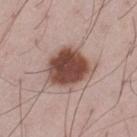The lesion was photographed on a routine skin check and not biopsied; there is no pathology result.
A male patient approximately 50 years of age.
On the leg.
A roughly 15 mm field-of-view crop from a total-body skin photograph.
An algorithmic analysis of the crop reported an eccentricity of roughly 0.6 and a symmetry-axis asymmetry near 0.2. The software also gave an average lesion color of about L≈48 a*≈20 b*≈24 (CIELAB), about 18 CIELAB-L* units darker than the surrounding skin, and a normalized lesion–skin contrast near 12. The software also gave internal color variation of about 5.5 on a 0–10 scale and peripheral color asymmetry of about 1.5.
Captured under white-light illumination.